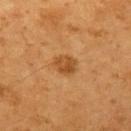Case summary:
• workup: imaged on a skin check; not biopsied